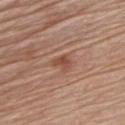<record>
<biopsy_status>not biopsied; imaged during a skin examination</biopsy_status>
<lighting>white-light</lighting>
<lesion_size>
  <long_diameter_mm_approx>2.5</long_diameter_mm_approx>
</lesion_size>
<image>
  <source>total-body photography crop</source>
  <field_of_view_mm>15</field_of_view_mm>
</image>
<site>chest</site>
<patient>
  <sex>male</sex>
  <age_approx>80</age_approx>
</patient>
<automated_metrics>
  <area_mm2_approx>4.0</area_mm2_approx>
  <eccentricity>0.65</eccentricity>
  <shape_asymmetry>0.4</shape_asymmetry>
  <cielab_L>49</cielab_L>
  <cielab_a>22</cielab_a>
  <cielab_b>29</cielab_b>
  <vs_skin_darker_L>8.0</vs_skin_darker_L>
  <vs_skin_contrast_norm>6.0</vs_skin_contrast_norm>
  <border_irregularity_0_10>3.5</border_irregularity_0_10>
  <color_variation_0_10>3.5</color_variation_0_10>
  <peripheral_color_asymmetry>1.0</peripheral_color_asymmetry>
</automated_metrics>
</record>